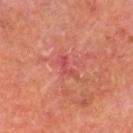This lesion was catalogued during total-body skin photography and was not selected for biopsy. A close-up tile cropped from a whole-body skin photograph, about 15 mm across. The lesion is located on the leg. A male subject aged around 65.